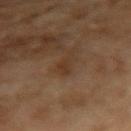Part of a total-body skin-imaging series; this lesion was reviewed on a skin check and was not flagged for biopsy. About 3 mm across. This image is a 15 mm lesion crop taken from a total-body photograph. On the right upper arm. A female subject aged approximately 60.